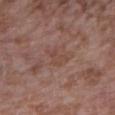biopsy_status: not biopsied; imaged during a skin examination
lesion_size:
  long_diameter_mm_approx: 2.5
site: right upper arm
lighting: white-light
image:
  source: total-body photography crop
  field_of_view_mm: 15
patient:
  sex: female
  age_approx: 70